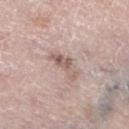| field | value |
|---|---|
| biopsy status | no biopsy performed (imaged during a skin exam) |
| anatomic site | the leg |
| automated lesion analysis | a lesion area of about 5.5 mm², an outline eccentricity of about 0.9 (0 = round, 1 = elongated), and a symmetry-axis asymmetry near 0.45; an average lesion color of about L≈59 a*≈17 b*≈23 (CIELAB) and about 10 CIELAB-L* units darker than the surrounding skin; a border-irregularity rating of about 5/10 and a peripheral color-asymmetry measure near 0.5; an automated nevus-likeness rating near 15 out of 100 and lesion-presence confidence of about 100/100 |
| image | ~15 mm tile from a whole-body skin photo |
| patient | female, aged around 65 |
| lighting | white-light |
| size | ~4 mm (longest diameter) |